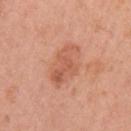Case summary:
– patient: female, aged 53–57
– size: about 5.5 mm
– body site: the left upper arm
– automated lesion analysis: a shape eccentricity near 0.75 and two-axis asymmetry of about 0.2; border irregularity of about 2 on a 0–10 scale, a color-variation rating of about 4.5/10, and a peripheral color-asymmetry measure near 1.5; an automated nevus-likeness rating near 0 out of 100 and a detector confidence of about 100 out of 100 that the crop contains a lesion
– imaging modality: ~15 mm tile from a whole-body skin photo
– illumination: white-light illumination